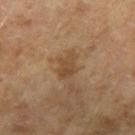The subject is a female aged 58–62. The lesion is on the right upper arm. The lesion's longest dimension is about 2.5 mm. A close-up tile cropped from a whole-body skin photograph, about 15 mm across.